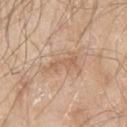- follow-up — total-body-photography surveillance lesion; no biopsy
- illumination — white-light illumination
- body site — the right upper arm
- automated lesion analysis — a classifier nevus-likeness of about 0/100 and a lesion-detection confidence of about 95/100
- imaging modality — ~15 mm crop, total-body skin-cancer survey
- patient — male, aged 63–67